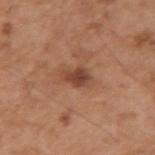Impression:
Captured during whole-body skin photography for melanoma surveillance; the lesion was not biopsied.
Context:
A male patient, roughly 55 years of age. From the right upper arm. A 15 mm close-up tile from a total-body photography series done for melanoma screening. Measured at roughly 3 mm in maximum diameter.